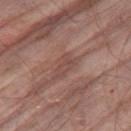Assessment: Captured during whole-body skin photography for melanoma surveillance; the lesion was not biopsied. Context: Cropped from a total-body skin-imaging series; the visible field is about 15 mm. On the right thigh. The tile uses white-light illumination. A male patient in their 80s.